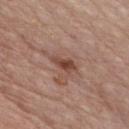No biopsy was performed on this lesion — it was imaged during a full skin examination and was not determined to be concerning. Cropped from a total-body skin-imaging series; the visible field is about 15 mm. A male patient, approximately 65 years of age. The lesion is on the chest. Longest diameter approximately 3.5 mm.